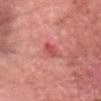| field | value |
|---|---|
| follow-up | imaged on a skin check; not biopsied |
| lighting | white-light illumination |
| patient | male, aged 48 to 52 |
| lesion size | about 2.5 mm |
| TBP lesion metrics | a classifier nevus-likeness of about 0/100 |
| location | the head or neck |
| image | 15 mm crop, total-body photography |